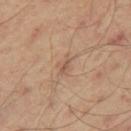Part of a total-body skin-imaging series; this lesion was reviewed on a skin check and was not flagged for biopsy.
A male patient aged around 50.
Automated tile analysis of the lesion measured a lesion area of about 3 mm² and an outline eccentricity of about 0.85 (0 = round, 1 = elongated). It also reported an average lesion color of about L≈54 a*≈18 b*≈29 (CIELAB), a lesion–skin lightness drop of about 7, and a normalized lesion–skin contrast near 5. It also reported an automated nevus-likeness rating near 0 out of 100 and lesion-presence confidence of about 100/100.
On the left leg.
The recorded lesion diameter is about 2.5 mm.
A 15 mm close-up tile from a total-body photography series done for melanoma screening.
Imaged with cross-polarized lighting.